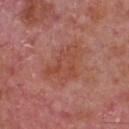Captured during whole-body skin photography for melanoma surveillance; the lesion was not biopsied. The total-body-photography lesion software estimated a lesion area of about 10 mm², a shape eccentricity near 0.75, and a shape-asymmetry score of about 0.6 (0 = symmetric). It also reported a mean CIELAB color near L≈48 a*≈27 b*≈30, a lesion–skin lightness drop of about 6, and a normalized lesion–skin contrast near 5.5. And it measured a detector confidence of about 100 out of 100 that the crop contains a lesion. Approximately 5 mm at its widest. The lesion is located on the front of the torso. Cropped from a total-body skin-imaging series; the visible field is about 15 mm. A male patient roughly 65 years of age. Captured under white-light illumination.